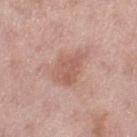Impression:
The lesion was tiled from a total-body skin photograph and was not biopsied.
Background:
A female subject, in their 50s. Cropped from a whole-body photographic skin survey; the tile spans about 15 mm. Approximately 4.5 mm at its widest. Imaged with white-light lighting. The lesion is on the left thigh.